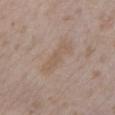Assessment: Part of a total-body skin-imaging series; this lesion was reviewed on a skin check and was not flagged for biopsy. Acquisition and patient details: Imaged with white-light lighting. A female patient, in their mid- to late 20s. The lesion is located on the left lower leg. A 15 mm close-up extracted from a 3D total-body photography capture. An algorithmic analysis of the crop reported a shape-asymmetry score of about 0.2 (0 = symmetric). The software also gave a border-irregularity rating of about 3.5/10 and radial color variation of about 0.5. Measured at roughly 5 mm in maximum diameter.